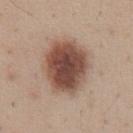Clinical impression:
Imaged during a routine full-body skin examination; the lesion was not biopsied and no histopathology is available.
Context:
The subject is a male aged around 35. A 15 mm close-up extracted from a 3D total-body photography capture. From the abdomen.